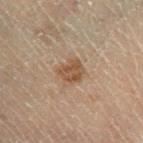The lesion is located on the leg. This is a cross-polarized tile. A 15 mm crop from a total-body photograph taken for skin-cancer surveillance. A male patient roughly 70 years of age. Approximately 3 mm at its widest.